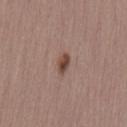Q: Where on the body is the lesion?
A: the lower back
Q: Illumination type?
A: white-light illumination
Q: What is the imaging modality?
A: ~15 mm crop, total-body skin-cancer survey
Q: Who is the patient?
A: female, roughly 45 years of age
Q: What is the lesion's diameter?
A: ≈2.5 mm